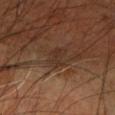notes: catalogued during a skin exam; not biopsied | TBP lesion metrics: a shape eccentricity near 0.7 and a symmetry-axis asymmetry near 0.3; about 5 CIELAB-L* units darker than the surrounding skin; an automated nevus-likeness rating near 0 out of 100 and a detector confidence of about 85 out of 100 that the crop contains a lesion | acquisition: total-body-photography crop, ~15 mm field of view | size: about 3 mm | anatomic site: the left forearm | patient: male, in their 60s | tile lighting: cross-polarized.